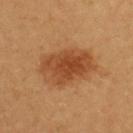Q: Was this lesion biopsied?
A: imaged on a skin check; not biopsied
Q: Automated lesion metrics?
A: a footprint of about 21 mm², an outline eccentricity of about 0.8 (0 = round, 1 = elongated), and a shape-asymmetry score of about 0.15 (0 = symmetric); a within-lesion color-variation index near 5/10 and peripheral color asymmetry of about 1.5; a classifier nevus-likeness of about 100/100 and lesion-presence confidence of about 100/100
Q: What is the imaging modality?
A: 15 mm crop, total-body photography
Q: What is the anatomic site?
A: the upper back
Q: Illumination type?
A: cross-polarized illumination
Q: What is the lesion's diameter?
A: ≈6.5 mm
Q: Who is the patient?
A: female, aged 58 to 62